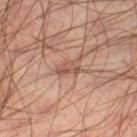  patient:
    sex: male
    age_approx: 45
  site: leg
  lesion_size:
    long_diameter_mm_approx: 3.0
  image:
    source: total-body photography crop
    field_of_view_mm: 15